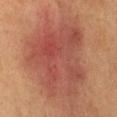workup = total-body-photography surveillance lesion; no biopsy
size = ≈9.5 mm
lighting = cross-polarized
image-analysis metrics = a footprint of about 50 mm² and a shape eccentricity near 0.65; an average lesion color of about L≈41 a*≈24 b*≈25 (CIELAB) and a lesion–skin lightness drop of about 8; a within-lesion color-variation index near 6/10; a nevus-likeness score of about 5/100 and a lesion-detection confidence of about 100/100
patient = female, aged 38 to 42
body site = the front of the torso
image = 15 mm crop, total-body photography